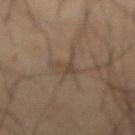Case summary:
• biopsy status — total-body-photography surveillance lesion; no biopsy
• patient — male, in their 70s
• tile lighting — cross-polarized
• diameter — about 3.5 mm
• image source — ~15 mm crop, total-body skin-cancer survey
• image-analysis metrics — a lesion area of about 3.5 mm² and a shape eccentricity near 0.8; an automated nevus-likeness rating near 0 out of 100 and a detector confidence of about 70 out of 100 that the crop contains a lesion
• location — the abdomen